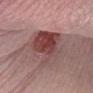Imaged during a routine full-body skin examination; the lesion was not biopsied and no histopathology is available.
Located on the arm.
Imaged with white-light lighting.
A 15 mm crop from a total-body photograph taken for skin-cancer surveillance.
Automated image analysis of the tile measured a footprint of about 22 mm² and an eccentricity of roughly 0.85. It also reported a lesion color around L≈44 a*≈23 b*≈20 in CIELAB and roughly 13 lightness units darker than nearby skin. And it measured border irregularity of about 3 on a 0–10 scale, a color-variation rating of about 10/10, and peripheral color asymmetry of about 3.5. It also reported an automated nevus-likeness rating near 70 out of 100 and lesion-presence confidence of about 65/100.
A male patient aged 58–62.